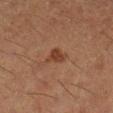Impression:
The lesion was photographed on a routine skin check and not biopsied; there is no pathology result.
Context:
Imaged with cross-polarized lighting. The lesion-visualizer software estimated a lesion area of about 4 mm². It also reported roughly 7 lightness units darker than nearby skin and a lesion-to-skin contrast of about 7.5 (normalized; higher = more distinct). The analysis additionally found lesion-presence confidence of about 100/100. A 15 mm crop from a total-body photograph taken for skin-cancer surveillance. A female subject, aged approximately 50. From the right lower leg.